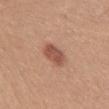Clinical impression:
Captured during whole-body skin photography for melanoma surveillance; the lesion was not biopsied.
Acquisition and patient details:
Cropped from a whole-body photographic skin survey; the tile spans about 15 mm. A female subject in their mid-50s. About 3.5 mm across. Captured under white-light illumination. On the abdomen.